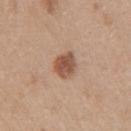{"biopsy_status": "not biopsied; imaged during a skin examination", "patient": {"sex": "female", "age_approx": 40}, "automated_metrics": {"area_mm2_approx": 6.0, "eccentricity": 0.65, "shape_asymmetry": 0.2, "nevus_likeness_0_100": 95, "lesion_detection_confidence_0_100": 100}, "site": "right upper arm", "image": {"source": "total-body photography crop", "field_of_view_mm": 15}, "lighting": "white-light"}